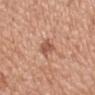Recorded during total-body skin imaging; not selected for excision or biopsy.